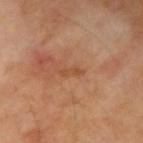A male patient, aged approximately 70. About 2.5 mm across. On the right upper arm. A 15 mm close-up extracted from a 3D total-body photography capture. Captured under cross-polarized illumination.